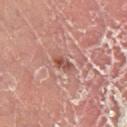| field | value |
|---|---|
| notes | no biopsy performed (imaged during a skin exam) |
| lesion diameter | ≈2.5 mm |
| anatomic site | the left lower leg |
| subject | male, in their mid-60s |
| acquisition | ~15 mm tile from a whole-body skin photo |
| TBP lesion metrics | a symmetry-axis asymmetry near 0.4; a border-irregularity index near 3.5/10, a color-variation rating of about 0/10, and radial color variation of about 0 |